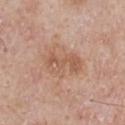| field | value |
|---|---|
| notes | no biopsy performed (imaged during a skin exam) |
| automated lesion analysis | an eccentricity of roughly 0.75 and a shape-asymmetry score of about 0.35 (0 = symmetric); a border-irregularity rating of about 5/10 and internal color variation of about 3.5 on a 0–10 scale; a classifier nevus-likeness of about 0/100 and a lesion-detection confidence of about 100/100 |
| body site | the front of the torso |
| patient | male, aged around 65 |
| image | total-body-photography crop, ~15 mm field of view |
| tile lighting | white-light illumination |
| diameter | about 5 mm |